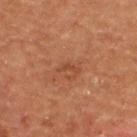* notes · no biopsy performed (imaged during a skin exam)
* body site · the back
* lighting · cross-polarized
* imaging modality · total-body-photography crop, ~15 mm field of view
* subject · male, roughly 55 years of age
* automated lesion analysis · border irregularity of about 6 on a 0–10 scale and a within-lesion color-variation index near 0/10